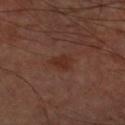The lesion was tiled from a total-body skin photograph and was not biopsied. The lesion is on the left upper arm. A male subject, aged approximately 60. This image is a 15 mm lesion crop taken from a total-body photograph. Automated image analysis of the tile measured an area of roughly 4.5 mm² and a shape eccentricity near 0.75. The software also gave a lesion color around L≈29 a*≈21 b*≈25 in CIELAB and roughly 6 lightness units darker than nearby skin. The analysis additionally found a border-irregularity rating of about 2.5/10 and radial color variation of about 0.5.